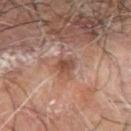Q: Was this lesion biopsied?
A: no biopsy performed (imaged during a skin exam)
Q: How was the tile lit?
A: cross-polarized
Q: What is the anatomic site?
A: the left forearm
Q: What is the imaging modality?
A: total-body-photography crop, ~15 mm field of view
Q: What is the lesion's diameter?
A: about 2.5 mm
Q: What are the patient's age and sex?
A: male, roughly 65 years of age